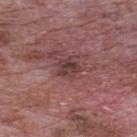Assessment: Imaged during a routine full-body skin examination; the lesion was not biopsied and no histopathology is available. Context: Captured under white-light illumination. Located on the upper back. The lesion's longest dimension is about 3 mm. The total-body-photography lesion software estimated border irregularity of about 3 on a 0–10 scale, internal color variation of about 4.5 on a 0–10 scale, and radial color variation of about 1.5. The subject is a male approximately 70 years of age. Cropped from a total-body skin-imaging series; the visible field is about 15 mm.